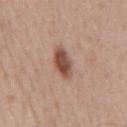* notes: total-body-photography surveillance lesion; no biopsy
* TBP lesion metrics: an area of roughly 7 mm² and two-axis asymmetry of about 0.25; a lesion color around L≈50 a*≈21 b*≈28 in CIELAB, roughly 14 lightness units darker than nearby skin, and a lesion-to-skin contrast of about 10 (normalized; higher = more distinct); border irregularity of about 2.5 on a 0–10 scale, internal color variation of about 4 on a 0–10 scale, and a peripheral color-asymmetry measure near 1; an automated nevus-likeness rating near 100 out of 100
* size: ~4 mm (longest diameter)
* body site: the mid back
* illumination: white-light
* subject: male, aged approximately 50
* imaging modality: ~15 mm crop, total-body skin-cancer survey